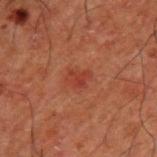Q: Was this lesion biopsied?
A: total-body-photography surveillance lesion; no biopsy
Q: How was the tile lit?
A: cross-polarized illumination
Q: How was this image acquired?
A: ~15 mm crop, total-body skin-cancer survey
Q: Automated lesion metrics?
A: an area of roughly 4 mm², an eccentricity of roughly 0.7, and a symmetry-axis asymmetry near 0.3; a lesion color around L≈30 a*≈25 b*≈25 in CIELAB, a lesion–skin lightness drop of about 5, and a lesion-to-skin contrast of about 5.5 (normalized; higher = more distinct); border irregularity of about 3 on a 0–10 scale, a color-variation rating of about 1.5/10, and radial color variation of about 0.5
Q: What is the anatomic site?
A: the upper back
Q: How large is the lesion?
A: ~2.5 mm (longest diameter)
Q: Who is the patient?
A: male, aged approximately 50